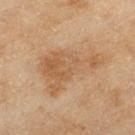Part of a total-body skin-imaging series; this lesion was reviewed on a skin check and was not flagged for biopsy. Measured at roughly 8 mm in maximum diameter. A lesion tile, about 15 mm wide, cut from a 3D total-body photograph. A female subject aged around 60. The lesion is on the left thigh. Captured under cross-polarized illumination. Automated tile analysis of the lesion measured a lesion–skin lightness drop of about 7 and a lesion-to-skin contrast of about 5.5 (normalized; higher = more distinct). It also reported a border-irregularity rating of about 6/10, a color-variation rating of about 3.5/10, and radial color variation of about 1.